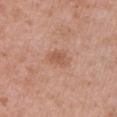Clinical impression: No biopsy was performed on this lesion — it was imaged during a full skin examination and was not determined to be concerning. Context: Captured under white-light illumination. Longest diameter approximately 3.5 mm. A close-up tile cropped from a whole-body skin photograph, about 15 mm across. A female patient aged approximately 50. The lesion is located on the arm.